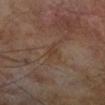Q: Was a biopsy performed?
A: catalogued during a skin exam; not biopsied
Q: Lesion size?
A: ≈3.5 mm
Q: What did automated image analysis measure?
A: an area of roughly 4 mm², a shape eccentricity near 0.85, and two-axis asymmetry of about 0.4; an average lesion color of about L≈37 a*≈16 b*≈26 (CIELAB) and a lesion–skin lightness drop of about 5; a border-irregularity rating of about 5/10, internal color variation of about 1 on a 0–10 scale, and radial color variation of about 0.5; a nevus-likeness score of about 0/100 and a lesion-detection confidence of about 95/100
Q: What kind of image is this?
A: total-body-photography crop, ~15 mm field of view
Q: What are the patient's age and sex?
A: male, aged around 65
Q: What lighting was used for the tile?
A: cross-polarized